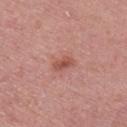The lesion was photographed on a routine skin check and not biopsied; there is no pathology result.
Cropped from a whole-body photographic skin survey; the tile spans about 15 mm.
Located on the right upper arm.
The lesion's longest dimension is about 2.5 mm.
The tile uses white-light illumination.
Automated image analysis of the tile measured a lesion color around L≈52 a*≈27 b*≈27 in CIELAB, a lesion–skin lightness drop of about 10, and a normalized lesion–skin contrast near 7. And it measured a peripheral color-asymmetry measure near 0.5. It also reported an automated nevus-likeness rating near 45 out of 100.
A male subject aged 53–57.